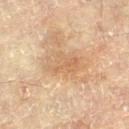follow-up = total-body-photography surveillance lesion; no biopsy
TBP lesion metrics = roughly 7 lightness units darker than nearby skin and a normalized border contrast of about 5
imaging modality = 15 mm crop, total-body photography
lesion diameter = about 5 mm
location = the right leg
tile lighting = cross-polarized
subject = female, about 80 years old